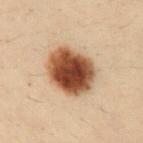Captured during whole-body skin photography for melanoma surveillance; the lesion was not biopsied.
An algorithmic analysis of the crop reported a lesion–skin lightness drop of about 20 and a normalized border contrast of about 15. And it measured border irregularity of about 1 on a 0–10 scale, internal color variation of about 6.5 on a 0–10 scale, and a peripheral color-asymmetry measure near 2. And it measured an automated nevus-likeness rating near 100 out of 100 and a lesion-detection confidence of about 100/100.
On the abdomen.
Longest diameter approximately 5 mm.
The tile uses cross-polarized illumination.
Cropped from a total-body skin-imaging series; the visible field is about 15 mm.
A male subject, in their 30s.